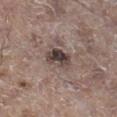The lesion was tiled from a total-body skin photograph and was not biopsied. Imaged with white-light lighting. A male subject aged approximately 65. The lesion is located on the right lower leg. Measured at roughly 3.5 mm in maximum diameter. A 15 mm close-up extracted from a 3D total-body photography capture. The total-body-photography lesion software estimated an area of roughly 7 mm² and two-axis asymmetry of about 0.2. It also reported a lesion–skin lightness drop of about 13 and a normalized border contrast of about 10.5. It also reported border irregularity of about 2 on a 0–10 scale, a color-variation rating of about 8/10, and peripheral color asymmetry of about 3. The analysis additionally found a nevus-likeness score of about 20/100 and lesion-presence confidence of about 100/100.